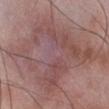workup: no biopsy performed (imaged during a skin exam) | acquisition: ~15 mm crop, total-body skin-cancer survey | lesion size: about 13.5 mm | location: the right lower leg | illumination: white-light | image-analysis metrics: an area of roughly 75 mm², an eccentricity of roughly 0.7, and a symmetry-axis asymmetry near 0.35; roughly 8 lightness units darker than nearby skin and a lesion-to-skin contrast of about 6 (normalized; higher = more distinct); border irregularity of about 6 on a 0–10 scale and peripheral color asymmetry of about 2; a classifier nevus-likeness of about 0/100 | subject: male, in their 50s.